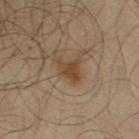Assessment: Part of a total-body skin-imaging series; this lesion was reviewed on a skin check and was not flagged for biopsy. Acquisition and patient details: An algorithmic analysis of the crop reported a shape eccentricity near 0.6. The analysis additionally found a nevus-likeness score of about 55/100 and a detector confidence of about 100 out of 100 that the crop contains a lesion. A region of skin cropped from a whole-body photographic capture, roughly 15 mm wide. A male subject, aged 63–67. Located on the right upper arm. About 3.5 mm across. Imaged with cross-polarized lighting.